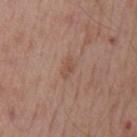illumination: white-light
imaging modality: ~15 mm tile from a whole-body skin photo
site: the mid back
TBP lesion metrics: a shape-asymmetry score of about 0.4 (0 = symmetric); a mean CIELAB color near L≈50 a*≈20 b*≈28, a lesion–skin lightness drop of about 7, and a normalized lesion–skin contrast near 5.5; a border-irregularity rating of about 3.5/10, a color-variation rating of about 0.5/10, and radial color variation of about 0.5; an automated nevus-likeness rating near 0 out of 100 and a detector confidence of about 100 out of 100 that the crop contains a lesion
subject: male, aged 53 to 57
lesion size: about 2.5 mm